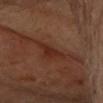Impression:
No biopsy was performed on this lesion — it was imaged during a full skin examination and was not determined to be concerning.
Acquisition and patient details:
From the head or neck. Cropped from a total-body skin-imaging series; the visible field is about 15 mm. A female subject aged 58 to 62. The lesion's longest dimension is about 4.5 mm. Captured under cross-polarized illumination.